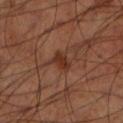Imaged during a routine full-body skin examination; the lesion was not biopsied and no histopathology is available. The lesion is on the left lower leg. A 15 mm crop from a total-body photograph taken for skin-cancer surveillance. Imaged with cross-polarized lighting. A male patient, about 70 years old. The recorded lesion diameter is about 2.5 mm. An algorithmic analysis of the crop reported an average lesion color of about L≈31 a*≈22 b*≈27 (CIELAB), about 8 CIELAB-L* units darker than the surrounding skin, and a lesion-to-skin contrast of about 8 (normalized; higher = more distinct). The analysis additionally found a classifier nevus-likeness of about 70/100 and lesion-presence confidence of about 100/100.